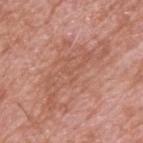{"biopsy_status": "not biopsied; imaged during a skin examination", "site": "back", "patient": {"sex": "male", "age_approx": 60}, "image": {"source": "total-body photography crop", "field_of_view_mm": 15}, "lesion_size": {"long_diameter_mm_approx": 10.0}}